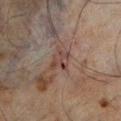Clinical summary: Longest diameter approximately 3 mm. This image is a 15 mm lesion crop taken from a total-body photograph. This is a cross-polarized tile. The lesion is located on the right lower leg. A male patient aged 63 to 67.